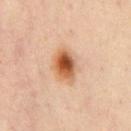Impression: Part of a total-body skin-imaging series; this lesion was reviewed on a skin check and was not flagged for biopsy. Image and clinical context: A 15 mm close-up tile from a total-body photography series done for melanoma screening. The lesion is located on the chest. A male subject, in their mid- to late 40s.